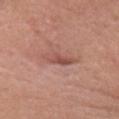This lesion was catalogued during total-body skin photography and was not selected for biopsy. The total-body-photography lesion software estimated a border-irregularity rating of about 4/10. A roughly 15 mm field-of-view crop from a total-body skin photograph. The lesion is on the head or neck. A female patient in their mid-70s. This is a white-light tile.